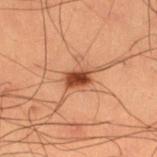follow-up: imaged on a skin check; not biopsied
patient: male, approximately 50 years of age
body site: the right thigh
lesion size: ~3.5 mm (longest diameter)
TBP lesion metrics: a nevus-likeness score of about 100/100 and a detector confidence of about 100 out of 100 that the crop contains a lesion
tile lighting: cross-polarized
image: ~15 mm tile from a whole-body skin photo